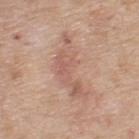biopsy_status: not biopsied; imaged during a skin examination
patient:
  sex: male
  age_approx: 70
image:
  source: total-body photography crop
  field_of_view_mm: 15
lesion_size:
  long_diameter_mm_approx: 7.5
site: back
automated_metrics:
  lesion_detection_confidence_0_100: 100
lighting: white-light